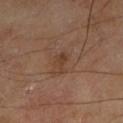notes: total-body-photography surveillance lesion; no biopsy | illumination: cross-polarized | location: the left lower leg | patient: male, aged 63 to 67 | automated lesion analysis: a mean CIELAB color near L≈39 a*≈18 b*≈29, roughly 7 lightness units darker than nearby skin, and a lesion-to-skin contrast of about 6.5 (normalized; higher = more distinct); border irregularity of about 4.5 on a 0–10 scale and a within-lesion color-variation index near 1/10 | imaging modality: 15 mm crop, total-body photography.